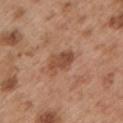biopsy_status: not biopsied; imaged during a skin examination
site: left upper arm
patient:
  sex: male
  age_approx: 55
lesion_size:
  long_diameter_mm_approx: 3.5
image:
  source: total-body photography crop
  field_of_view_mm: 15
lighting: white-light